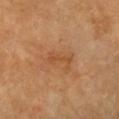The lesion was tiled from a total-body skin photograph and was not biopsied.